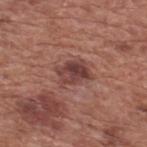The lesion was tiled from a total-body skin photograph and was not biopsied.
Imaged with white-light lighting.
Located on the upper back.
A male patient aged approximately 75.
Cropped from a whole-body photographic skin survey; the tile spans about 15 mm.